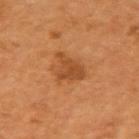Assessment:
No biopsy was performed on this lesion — it was imaged during a full skin examination and was not determined to be concerning.
Acquisition and patient details:
Located on the right upper arm. Captured under cross-polarized illumination. The patient is a male in their mid-50s. A 15 mm crop from a total-body photograph taken for skin-cancer surveillance.